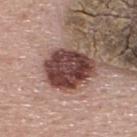acquisition = ~15 mm tile from a whole-body skin photo
size = ~6 mm (longest diameter)
anatomic site = the upper back
patient = male, aged 53 to 57
lighting = white-light